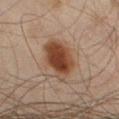No biopsy was performed on this lesion — it was imaged during a full skin examination and was not determined to be concerning.
Cropped from a total-body skin-imaging series; the visible field is about 15 mm.
Measured at roughly 5.5 mm in maximum diameter.
From the left thigh.
A male subject, approximately 45 years of age.